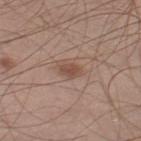The lesion was tiled from a total-body skin photograph and was not biopsied. A male patient in their mid-50s. A roughly 15 mm field-of-view crop from a total-body skin photograph. The tile uses white-light illumination. On the right thigh. About 2.5 mm across.